An algorithmic analysis of the crop reported a footprint of about 31 mm² and an eccentricity of roughly 0.7. And it measured a detector confidence of about 100 out of 100 that the crop contains a lesion, a female subject aged 38–42, measured at roughly 8 mm in maximum diameter, located on the right lower leg, this is a white-light tile, this image is a 15 mm lesion crop taken from a total-body photograph: 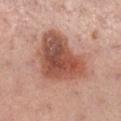Conclusion: On biopsy, histopathology showed a benign lesion: seborrheic keratosis.